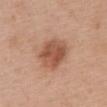notes = total-body-photography surveillance lesion; no biopsy | lighting = white-light illumination | lesion size = about 5 mm | subject = female, in their 60s | image source = total-body-photography crop, ~15 mm field of view | site = the upper back.